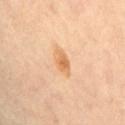workup = imaged on a skin check; not biopsied
body site = the abdomen
lesion diameter = ~3 mm (longest diameter)
patient = female, roughly 65 years of age
tile lighting = cross-polarized illumination
image source = 15 mm crop, total-body photography
automated metrics = a lesion area of about 4 mm² and two-axis asymmetry of about 0.2; a nevus-likeness score of about 60/100 and lesion-presence confidence of about 100/100